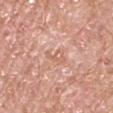Q: What is the anatomic site?
A: the right upper arm
Q: What is the imaging modality?
A: 15 mm crop, total-body photography
Q: What are the patient's age and sex?
A: male, aged 63 to 67
Q: What lighting was used for the tile?
A: white-light illumination
Q: What did automated image analysis measure?
A: a footprint of about 3.5 mm², a shape eccentricity near 0.75, and a shape-asymmetry score of about 0.5 (0 = symmetric); a mean CIELAB color near L≈63 a*≈23 b*≈31, about 7 CIELAB-L* units darker than the surrounding skin, and a lesion-to-skin contrast of about 5 (normalized; higher = more distinct); a nevus-likeness score of about 0/100
Q: Lesion size?
A: about 2.5 mm